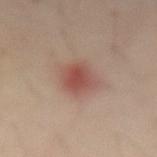Clinical impression:
Part of a total-body skin-imaging series; this lesion was reviewed on a skin check and was not flagged for biopsy.